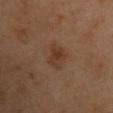Imaged during a routine full-body skin examination; the lesion was not biopsied and no histopathology is available. Longest diameter approximately 3.5 mm. This is a cross-polarized tile. The patient is a male in their 70s. A lesion tile, about 15 mm wide, cut from a 3D total-body photograph. Located on the chest.